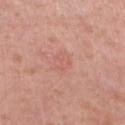size — ≈3 mm | image source — 15 mm crop, total-body photography | subject — female, in their 40s | image-analysis metrics — an eccentricity of roughly 0.75 and a symmetry-axis asymmetry near 0.25; a lesion-detection confidence of about 100/100 | illumination — white-light | body site — the left lower leg.